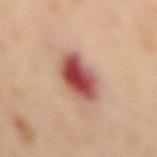Case summary:
– follow-up — no biopsy performed (imaged during a skin exam)
– lesion diameter — about 4.5 mm
– subject — female, roughly 55 years of age
– imaging modality — total-body-photography crop, ~15 mm field of view
– tile lighting — cross-polarized
– image-analysis metrics — a mean CIELAB color near L≈52 a*≈29 b*≈26, a lesion–skin lightness drop of about 18, and a lesion-to-skin contrast of about 12 (normalized; higher = more distinct); a classifier nevus-likeness of about 0/100 and a detector confidence of about 100 out of 100 that the crop contains a lesion
– location — the back